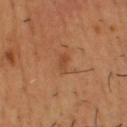| feature | finding |
|---|---|
| notes | catalogued during a skin exam; not biopsied |
| automated lesion analysis | a within-lesion color-variation index near 1/10 |
| site | the head or neck |
| size | ~3 mm (longest diameter) |
| patient | male, aged around 55 |
| image source | 15 mm crop, total-body photography |
| tile lighting | cross-polarized |